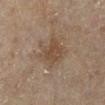Findings:
- lesion diameter · ~4 mm (longest diameter)
- TBP lesion metrics · a lesion area of about 11 mm², a shape eccentricity near 0.45, and two-axis asymmetry of about 0.2; about 7 CIELAB-L* units darker than the surrounding skin and a normalized lesion–skin contrast near 6; a border-irregularity index near 2.5/10, a within-lesion color-variation index near 3/10, and a peripheral color-asymmetry measure near 1
- anatomic site · the leg
- tile lighting · cross-polarized
- patient · female, about 60 years old
- imaging modality · total-body-photography crop, ~15 mm field of view